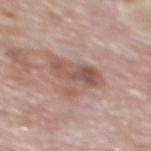Imaged during a routine full-body skin examination; the lesion was not biopsied and no histopathology is available. A 15 mm crop from a total-body photograph taken for skin-cancer surveillance. Longest diameter approximately 5 mm. The tile uses white-light illumination. The patient is a male aged 73–77. On the mid back.